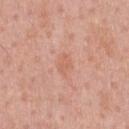Clinical impression: No biopsy was performed on this lesion — it was imaged during a full skin examination and was not determined to be concerning. Acquisition and patient details: A male patient aged 58–62. Imaged with white-light lighting. The lesion is located on the front of the torso. Cropped from a total-body skin-imaging series; the visible field is about 15 mm.